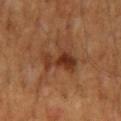workup — imaged on a skin check; not biopsied | image source — ~15 mm crop, total-body skin-cancer survey | tile lighting — cross-polarized illumination | patient — male, aged 58 to 62 | diameter — ~4.5 mm (longest diameter) | image-analysis metrics — a footprint of about 10 mm² and a symmetry-axis asymmetry near 0.45 | body site — the abdomen.